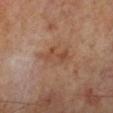Imaged during a routine full-body skin examination; the lesion was not biopsied and no histopathology is available.
This image is a 15 mm lesion crop taken from a total-body photograph.
The lesion is located on the left lower leg.
The subject is a male aged around 70.